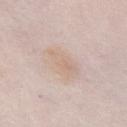Clinical impression: No biopsy was performed on this lesion — it was imaged during a full skin examination and was not determined to be concerning. Acquisition and patient details: The lesion is on the chest. A female patient, in their mid-60s. A 15 mm crop from a total-body photograph taken for skin-cancer surveillance.